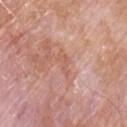Captured during whole-body skin photography for melanoma surveillance; the lesion was not biopsied.
Automated image analysis of the tile measured an area of roughly 3 mm² and a symmetry-axis asymmetry near 0.45.
The recorded lesion diameter is about 3 mm.
A lesion tile, about 15 mm wide, cut from a 3D total-body photograph.
A male patient, aged around 60.
On the right upper arm.
Captured under white-light illumination.